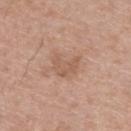This lesion was catalogued during total-body skin photography and was not selected for biopsy. The tile uses white-light illumination. The patient is a male aged approximately 40. The lesion-visualizer software estimated a shape eccentricity near 0.55 and a symmetry-axis asymmetry near 0.5. And it measured border irregularity of about 6 on a 0–10 scale, internal color variation of about 2 on a 0–10 scale, and radial color variation of about 0.5. The analysis additionally found a classifier nevus-likeness of about 0/100 and a detector confidence of about 100 out of 100 that the crop contains a lesion. A region of skin cropped from a whole-body photographic capture, roughly 15 mm wide. The recorded lesion diameter is about 3 mm. On the upper back.